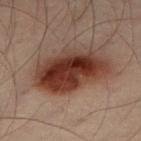Clinical impression: Imaged during a routine full-body skin examination; the lesion was not biopsied and no histopathology is available. Image and clinical context: Located on the left leg. The patient is a male approximately 50 years of age. Automated image analysis of the tile measured border irregularity of about 2 on a 0–10 scale and peripheral color asymmetry of about 2.5. The analysis additionally found a nevus-likeness score of about 100/100. This is a cross-polarized tile. Longest diameter approximately 8 mm. A 15 mm close-up tile from a total-body photography series done for melanoma screening.